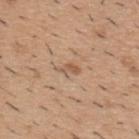Impression:
No biopsy was performed on this lesion — it was imaged during a full skin examination and was not determined to be concerning.
Image and clinical context:
An algorithmic analysis of the crop reported a lesion–skin lightness drop of about 8 and a lesion-to-skin contrast of about 6 (normalized; higher = more distinct). The analysis additionally found a border-irregularity index near 4/10 and peripheral color asymmetry of about 0. The analysis additionally found lesion-presence confidence of about 100/100. A male patient, approximately 40 years of age. The lesion is on the upper back. Cropped from a whole-body photographic skin survey; the tile spans about 15 mm. Approximately 3 mm at its widest. The tile uses white-light illumination.